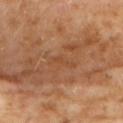Q: Was a biopsy performed?
A: no biopsy performed (imaged during a skin exam)
Q: What did automated image analysis measure?
A: an area of roughly 2.5 mm², an eccentricity of roughly 0.95, and a symmetry-axis asymmetry near 0.5; a border-irregularity rating of about 6.5/10, a color-variation rating of about 0/10, and radial color variation of about 0
Q: Where on the body is the lesion?
A: the upper back
Q: What is the lesion's diameter?
A: ≈3.5 mm
Q: How was this image acquired?
A: total-body-photography crop, ~15 mm field of view
Q: What lighting was used for the tile?
A: cross-polarized illumination
Q: Who is the patient?
A: female, approximately 60 years of age